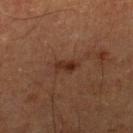{"site": "right lower leg", "lesion_size": {"long_diameter_mm_approx": 2.5}, "image": {"source": "total-body photography crop", "field_of_view_mm": 15}, "patient": {"sex": "male", "age_approx": 75}, "lighting": "cross-polarized"}